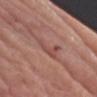Recorded during total-body skin imaging; not selected for excision or biopsy. A lesion tile, about 15 mm wide, cut from a 3D total-body photograph. The lesion is on the arm. A male patient about 85 years old or older.